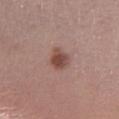| field | value |
|---|---|
| workup | no biopsy performed (imaged during a skin exam) |
| anatomic site | the left lower leg |
| imaging modality | total-body-photography crop, ~15 mm field of view |
| subject | female, roughly 65 years of age |
| lesion diameter | about 2.5 mm |